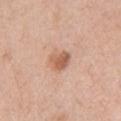Q: Is there a histopathology result?
A: catalogued during a skin exam; not biopsied
Q: Illumination type?
A: white-light illumination
Q: Patient demographics?
A: male, aged 68–72
Q: Lesion location?
A: the left upper arm
Q: Lesion size?
A: ~2.5 mm (longest diameter)
Q: How was this image acquired?
A: ~15 mm tile from a whole-body skin photo